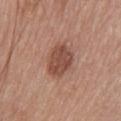biopsy status = no biopsy performed (imaged during a skin exam)